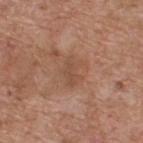Imaged during a routine full-body skin examination; the lesion was not biopsied and no histopathology is available. On the back. The recorded lesion diameter is about 3.5 mm. The patient is a male aged around 60. A 15 mm crop from a total-body photograph taken for skin-cancer surveillance. Captured under white-light illumination.